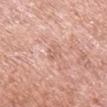| feature | finding |
|---|---|
| notes | total-body-photography surveillance lesion; no biopsy |
| body site | the right upper arm |
| patient | male, about 70 years old |
| acquisition | ~15 mm crop, total-body skin-cancer survey |